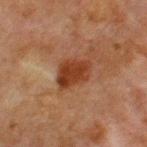{
  "biopsy_status": "not biopsied; imaged during a skin examination",
  "site": "chest",
  "lesion_size": {
    "long_diameter_mm_approx": 4.0
  },
  "patient": {
    "sex": "male",
    "age_approx": 65
  },
  "image": {
    "source": "total-body photography crop",
    "field_of_view_mm": 15
  },
  "automated_metrics": {
    "nevus_likeness_0_100": 90,
    "lesion_detection_confidence_0_100": 100
  }
}